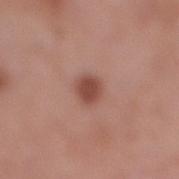workup = total-body-photography surveillance lesion; no biopsy
anatomic site = the right lower leg
patient = female, aged 53 to 57
image source = ~15 mm tile from a whole-body skin photo